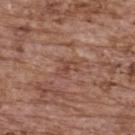notes=total-body-photography surveillance lesion; no biopsy | imaging modality=15 mm crop, total-body photography | subject=female, aged around 65 | location=the upper back.